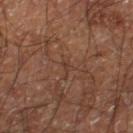Assessment: No biopsy was performed on this lesion — it was imaged during a full skin examination and was not determined to be concerning. Background: A 15 mm close-up extracted from a 3D total-body photography capture. A male patient aged 58 to 62. From the leg.